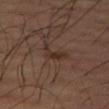No biopsy was performed on this lesion — it was imaged during a full skin examination and was not determined to be concerning. A lesion tile, about 15 mm wide, cut from a 3D total-body photograph. A male patient, aged 63 to 67. On the leg. The recorded lesion diameter is about 3 mm. An algorithmic analysis of the crop reported an average lesion color of about L≈30 a*≈16 b*≈23 (CIELAB), a lesion–skin lightness drop of about 7, and a lesion-to-skin contrast of about 7 (normalized; higher = more distinct). It also reported an automated nevus-likeness rating near 0 out of 100.